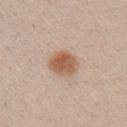Findings:
* biopsy status — total-body-photography surveillance lesion; no biopsy
* illumination — white-light
* anatomic site — the right upper arm
* diameter — ≈3.5 mm
* acquisition — total-body-photography crop, ~15 mm field of view
* patient — female, about 40 years old